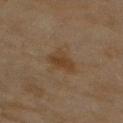Impression: The lesion was photographed on a routine skin check and not biopsied; there is no pathology result. Image and clinical context: A region of skin cropped from a whole-body photographic capture, roughly 15 mm wide. On the right upper arm. A female patient roughly 60 years of age.